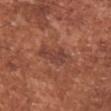Part of a total-body skin-imaging series; this lesion was reviewed on a skin check and was not flagged for biopsy.
A male patient, aged 43 to 47.
A 15 mm close-up extracted from a 3D total-body photography capture.
The lesion is located on the front of the torso.